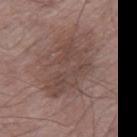<case>
  <biopsy_status>not biopsied; imaged during a skin examination</biopsy_status>
  <automated_metrics>
    <vs_skin_darker_L>7.0</vs_skin_darker_L>
    <border_irregularity_0_10>7.5</border_irregularity_0_10>
    <color_variation_0_10>2.5</color_variation_0_10>
    <peripheral_color_asymmetry>1.0</peripheral_color_asymmetry>
    <nevus_likeness_0_100>0</nevus_likeness_0_100>
  </automated_metrics>
  <site>right thigh</site>
  <patient>
    <sex>male</sex>
    <age_approx>55</age_approx>
  </patient>
  <image>
    <source>total-body photography crop</source>
    <field_of_view_mm>15</field_of_view_mm>
  </image>
  <lesion_size>
    <long_diameter_mm_approx>7.0</long_diameter_mm_approx>
  </lesion_size>
</case>